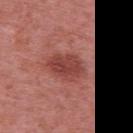Findings:
– follow-up · catalogued during a skin exam; not biopsied
– patient · female, in their 40s
– imaging modality · ~15 mm crop, total-body skin-cancer survey
– anatomic site · the upper back
– lesion size · about 4.5 mm
– automated metrics · an area of roughly 10 mm² and two-axis asymmetry of about 0.15; a lesion color around L≈44 a*≈29 b*≈26 in CIELAB and a lesion-to-skin contrast of about 7.5 (normalized; higher = more distinct); a nevus-likeness score of about 95/100